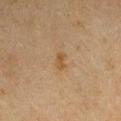Findings:
- workup: no biopsy performed (imaged during a skin exam)
- site: the right upper arm
- image-analysis metrics: a lesion area of about 3 mm² and an eccentricity of roughly 0.85; a lesion color around L≈41 a*≈14 b*≈31 in CIELAB and a lesion–skin lightness drop of about 6; border irregularity of about 3 on a 0–10 scale and internal color variation of about 0 on a 0–10 scale; a classifier nevus-likeness of about 5/100 and a lesion-detection confidence of about 100/100
- diameter: ≈2.5 mm
- image: ~15 mm crop, total-body skin-cancer survey
- patient: male, aged 63–67